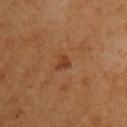follow-up: no biopsy performed (imaged during a skin exam) | body site: the right upper arm | diameter: about 2 mm | tile lighting: cross-polarized | subject: female, aged around 55 | acquisition: total-body-photography crop, ~15 mm field of view | image-analysis metrics: a footprint of about 2.5 mm² and a shape eccentricity near 0.65; about 9 CIELAB-L* units darker than the surrounding skin and a normalized border contrast of about 7; a border-irregularity index near 3.5/10 and a peripheral color-asymmetry measure near 0.5.